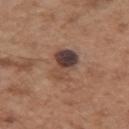The lesion was tiled from a total-body skin photograph and was not biopsied.
Measured at roughly 4.5 mm in maximum diameter.
This is a white-light tile.
Located on the left upper arm.
A 15 mm crop from a total-body photograph taken for skin-cancer surveillance.
A male subject, aged approximately 55.